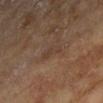Impression:
This lesion was catalogued during total-body skin photography and was not selected for biopsy.